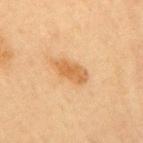Notes:
- notes — no biopsy performed (imaged during a skin exam)
- lighting — cross-polarized
- image source — ~15 mm tile from a whole-body skin photo
- location — the mid back
- patient — female, roughly 50 years of age
- TBP lesion metrics — a mean CIELAB color near L≈53 a*≈19 b*≈38 and a lesion-to-skin contrast of about 7.5 (normalized; higher = more distinct); a border-irregularity index near 2.5/10, a color-variation rating of about 2.5/10, and radial color variation of about 1; an automated nevus-likeness rating near 80 out of 100